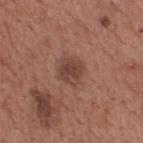tile lighting: white-light | TBP lesion metrics: a footprint of about 7 mm², a shape eccentricity near 0.45, and a shape-asymmetry score of about 0.15 (0 = symmetric); a border-irregularity rating of about 1.5/10, internal color variation of about 2.5 on a 0–10 scale, and a peripheral color-asymmetry measure near 1 | acquisition: ~15 mm crop, total-body skin-cancer survey | subject: male, roughly 65 years of age | body site: the upper back | size: about 3 mm.